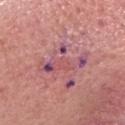{
  "lighting": "white-light",
  "patient": {
    "sex": "female",
    "age_approx": 40
  },
  "lesion_size": {
    "long_diameter_mm_approx": 6.0
  },
  "image": {
    "source": "total-body photography crop",
    "field_of_view_mm": 15
  },
  "site": "head or neck",
  "diagnosis": {
    "histopathology": "squamous cell carcinoma in situ",
    "malignancy": "malignant",
    "taxonomic_path": [
      "Malignant",
      "Malignant epidermal proliferations",
      "Squamous cell carcinoma in situ"
    ]
  }
}